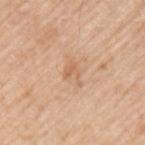anatomic site: the arm | diameter: ≈2.5 mm | image source: total-body-photography crop, ~15 mm field of view | automated lesion analysis: an outline eccentricity of about 0.6 (0 = round, 1 = elongated) and a shape-asymmetry score of about 0.65 (0 = symmetric); a color-variation rating of about 0.5/10 | tile lighting: white-light | subject: male, in their mid-50s.